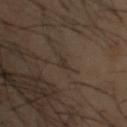Part of a total-body skin-imaging series; this lesion was reviewed on a skin check and was not flagged for biopsy. Automated image analysis of the tile measured an eccentricity of roughly 0.65 and a symmetry-axis asymmetry near 0.5. And it measured a mean CIELAB color near L≈28 a*≈9 b*≈19, roughly 5 lightness units darker than nearby skin, and a normalized lesion–skin contrast near 5.5. And it measured a border-irregularity index near 4/10. A 15 mm crop from a total-body photograph taken for skin-cancer surveillance. The tile uses cross-polarized illumination. A male patient, in their mid-40s. Located on the head or neck.